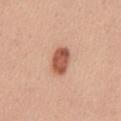The lesion was photographed on a routine skin check and not biopsied; there is no pathology result. Automated tile analysis of the lesion measured a border-irregularity rating of about 1.5/10. A female subject about 45 years old. From the mid back. This image is a 15 mm lesion crop taken from a total-body photograph. Captured under white-light illumination.